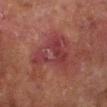{"biopsy_status": "not biopsied; imaged during a skin examination", "site": "left lower leg", "image": {"source": "total-body photography crop", "field_of_view_mm": 15}, "lesion_size": {"long_diameter_mm_approx": 5.5}, "patient": {"sex": "male", "age_approx": 70}, "lighting": "cross-polarized"}